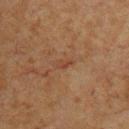{"biopsy_status": "not biopsied; imaged during a skin examination", "lesion_size": {"long_diameter_mm_approx": 2.5}, "image": {"source": "total-body photography crop", "field_of_view_mm": 15}, "site": "chest", "patient": {"sex": "male", "age_approx": 65}, "lighting": "cross-polarized"}